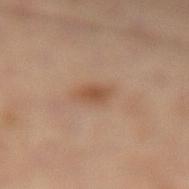location: the leg | subject: male, aged approximately 50 | lesion diameter: ~3 mm (longest diameter) | imaging modality: ~15 mm tile from a whole-body skin photo.